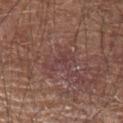Imaged during a routine full-body skin examination; the lesion was not biopsied and no histopathology is available.